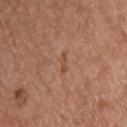Case summary:
- workup · catalogued during a skin exam; not biopsied
- imaging modality · ~15 mm crop, total-body skin-cancer survey
- anatomic site · the upper back
- patient · male, approximately 55 years of age